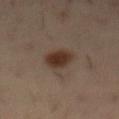The lesion was photographed on a routine skin check and not biopsied; there is no pathology result. An algorithmic analysis of the crop reported an automated nevus-likeness rating near 100 out of 100. This image is a 15 mm lesion crop taken from a total-body photograph. The lesion is on the mid back. A male subject in their mid- to late 50s. Approximately 3.5 mm at its widest. Imaged with cross-polarized lighting.